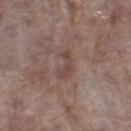Clinical summary: The subject is a female roughly 85 years of age or older. About 4 mm across. Cropped from a total-body skin-imaging series; the visible field is about 15 mm. The lesion-visualizer software estimated a lesion color around L≈45 a*≈17 b*≈22 in CIELAB and roughly 7 lightness units darker than nearby skin. The analysis additionally found a within-lesion color-variation index near 1/10 and a peripheral color-asymmetry measure near 0. The tile uses white-light illumination. The lesion is located on the left thigh.